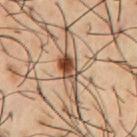Captured during whole-body skin photography for melanoma surveillance; the lesion was not biopsied. A region of skin cropped from a whole-body photographic capture, roughly 15 mm wide. The total-body-photography lesion software estimated a footprint of about 6 mm² and a shape eccentricity near 0.85. The analysis additionally found a mean CIELAB color near L≈46 a*≈19 b*≈31 and a lesion–skin lightness drop of about 17. The analysis additionally found border irregularity of about 3.5 on a 0–10 scale, internal color variation of about 10 on a 0–10 scale, and radial color variation of about 3.5. On the chest. The recorded lesion diameter is about 4 mm. The patient is a male aged around 55.